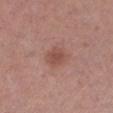Clinical impression:
The lesion was photographed on a routine skin check and not biopsied; there is no pathology result.
Context:
A 15 mm close-up tile from a total-body photography series done for melanoma screening. A female subject, aged 28–32. From the left lower leg. The tile uses white-light illumination.